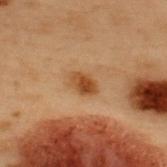Findings:
• biopsy status · imaged on a skin check; not biopsied
• lesion size · ~3 mm (longest diameter)
• site · the upper back
• subject · male, approximately 55 years of age
• illumination · cross-polarized illumination
• image · 15 mm crop, total-body photography